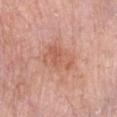Q: Was this lesion biopsied?
A: catalogued during a skin exam; not biopsied
Q: Where on the body is the lesion?
A: the chest
Q: Lesion size?
A: ≈4.5 mm
Q: What kind of image is this?
A: 15 mm crop, total-body photography
Q: How was the tile lit?
A: white-light
Q: Who is the patient?
A: female, aged around 65